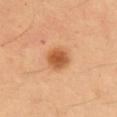No biopsy was performed on this lesion — it was imaged during a full skin examination and was not determined to be concerning. On the chest. Captured under cross-polarized illumination. A male subject, approximately 55 years of age. The recorded lesion diameter is about 3.5 mm. A region of skin cropped from a whole-body photographic capture, roughly 15 mm wide.